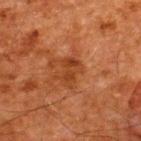{
  "biopsy_status": "not biopsied; imaged during a skin examination",
  "patient": {
    "sex": "male",
    "age_approx": 60
  },
  "site": "upper back",
  "image": {
    "source": "total-body photography crop",
    "field_of_view_mm": 15
  }
}